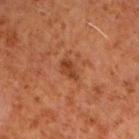Q: Was a biopsy performed?
A: no biopsy performed (imaged during a skin exam)
Q: What is the lesion's diameter?
A: ≈2.5 mm
Q: Lesion location?
A: the right forearm
Q: How was this image acquired?
A: ~15 mm crop, total-body skin-cancer survey
Q: Who is the patient?
A: male, in their 60s
Q: What did automated image analysis measure?
A: a lesion–skin lightness drop of about 9 and a lesion-to-skin contrast of about 7 (normalized; higher = more distinct); a nevus-likeness score of about 50/100 and a detector confidence of about 100 out of 100 that the crop contains a lesion